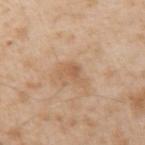Case summary:
* workup: catalogued during a skin exam; not biopsied
* diameter: ~2.5 mm (longest diameter)
* imaging modality: 15 mm crop, total-body photography
* location: the left upper arm
* automated metrics: border irregularity of about 5 on a 0–10 scale and a color-variation rating of about 1.5/10; a classifier nevus-likeness of about 0/100 and lesion-presence confidence of about 100/100
* patient: male, in their mid- to late 40s
* tile lighting: white-light